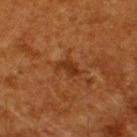workup = imaged on a skin check; not biopsied | patient = male, aged 58–62 | tile lighting = cross-polarized illumination | location = the back | image source = 15 mm crop, total-body photography | diameter = ≈3 mm | TBP lesion metrics = an average lesion color of about L≈32 a*≈24 b*≈35 (CIELAB), about 8 CIELAB-L* units darker than the surrounding skin, and a normalized border contrast of about 7.5; internal color variation of about 1.5 on a 0–10 scale and a peripheral color-asymmetry measure near 0.5; a nevus-likeness score of about 0/100 and lesion-presence confidence of about 100/100.